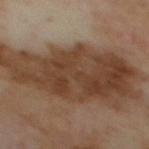Captured during whole-body skin photography for melanoma surveillance; the lesion was not biopsied. Approximately 13.5 mm at its widest. A 15 mm close-up tile from a total-body photography series done for melanoma screening. This is a cross-polarized tile. The lesion is on the mid back. A male subject aged around 70.